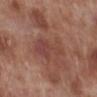Acquisition and patient details: The patient is a male in their 70s. This image is a 15 mm lesion crop taken from a total-body photograph. The lesion is on the right lower leg.